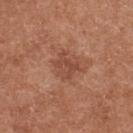Part of a total-body skin-imaging series; this lesion was reviewed on a skin check and was not flagged for biopsy.
Captured under white-light illumination.
Approximately 3 mm at its widest.
A close-up tile cropped from a whole-body skin photograph, about 15 mm across.
From the upper back.
Automated tile analysis of the lesion measured a lesion color around L≈47 a*≈25 b*≈30 in CIELAB and a normalized lesion–skin contrast near 6.
A female patient, aged 38–42.